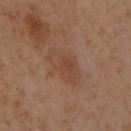Notes:
• follow-up: catalogued during a skin exam; not biopsied
• acquisition: ~15 mm crop, total-body skin-cancer survey
• TBP lesion metrics: a footprint of about 11 mm² and an outline eccentricity of about 0.85 (0 = round, 1 = elongated); border irregularity of about 3 on a 0–10 scale, a within-lesion color-variation index near 3/10, and a peripheral color-asymmetry measure near 1; an automated nevus-likeness rating near 0 out of 100 and a lesion-detection confidence of about 100/100
• anatomic site: the right lower leg
• lesion diameter: about 5 mm
• subject: female, in their mid- to late 50s
• tile lighting: cross-polarized illumination